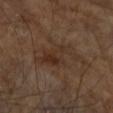Impression: No biopsy was performed on this lesion — it was imaged during a full skin examination and was not determined to be concerning. Background: Imaged with cross-polarized lighting. The patient is a male in their mid-80s or older. From the right forearm. A 15 mm close-up extracted from a 3D total-body photography capture. Measured at roughly 6 mm in maximum diameter.